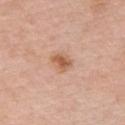follow-up = imaged on a skin check; not biopsied
lesion size = ~2.5 mm (longest diameter)
image-analysis metrics = internal color variation of about 3 on a 0–10 scale and a peripheral color-asymmetry measure near 1
illumination = white-light
subject = male, aged approximately 60
body site = the chest
image = ~15 mm tile from a whole-body skin photo